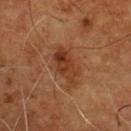Recorded during total-body skin imaging; not selected for excision or biopsy.